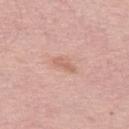Recorded during total-body skin imaging; not selected for excision or biopsy. The lesion is on the left thigh. A region of skin cropped from a whole-body photographic capture, roughly 15 mm wide. The subject is a female in their mid- to late 60s.